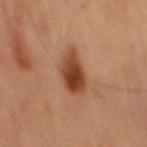{"biopsy_status": "not biopsied; imaged during a skin examination", "patient": {"sex": "male", "age_approx": 65}, "lighting": "cross-polarized", "lesion_size": {"long_diameter_mm_approx": 5.0}, "site": "abdomen", "automated_metrics": {"eccentricity": 0.85, "cielab_L": 33, "cielab_a": 20, "cielab_b": 27, "vs_skin_darker_L": 11.0, "vs_skin_contrast_norm": 10.5, "border_irregularity_0_10": 2.5, "peripheral_color_asymmetry": 2.0, "lesion_detection_confidence_0_100": 100}, "image": {"source": "total-body photography crop", "field_of_view_mm": 15}}